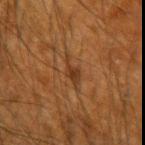The lesion was tiled from a total-body skin photograph and was not biopsied. Imaged with cross-polarized lighting. A male subject, about 65 years old. From the right forearm. The lesion's longest dimension is about 3 mm. A lesion tile, about 15 mm wide, cut from a 3D total-body photograph.